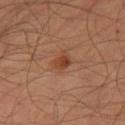Context: About 2 mm across. Imaged with cross-polarized lighting. The lesion is located on the right thigh. A male subject aged 53 to 57. A roughly 15 mm field-of-view crop from a total-body skin photograph.